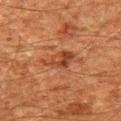<tbp_lesion>
  <biopsy_status>not biopsied; imaged during a skin examination</biopsy_status>
</tbp_lesion>